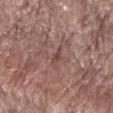Case summary:
* notes: catalogued during a skin exam; not biopsied
* imaging modality: 15 mm crop, total-body photography
* subject: male, aged 68 to 72
* location: the right forearm